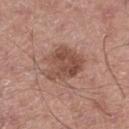Acquisition and patient details: The lesion is located on the leg. Captured under white-light illumination. About 4.5 mm across. Cropped from a whole-body photographic skin survey; the tile spans about 15 mm. The patient is a male aged 53–57.